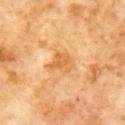Findings:
• notes · no biopsy performed (imaged during a skin exam)
• anatomic site · the front of the torso
• automated metrics · a footprint of about 6 mm², an outline eccentricity of about 0.4 (0 = round, 1 = elongated), and a shape-asymmetry score of about 0.3 (0 = symmetric); about 9 CIELAB-L* units darker than the surrounding skin; a border-irregularity index near 3/10, a within-lesion color-variation index near 2.5/10, and radial color variation of about 1; an automated nevus-likeness rating near 0 out of 100 and a detector confidence of about 100 out of 100 that the crop contains a lesion
• acquisition · total-body-photography crop, ~15 mm field of view
• patient · male, aged 58–62
• tile lighting · cross-polarized
• diameter · about 3 mm